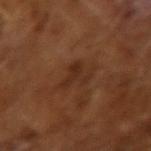This lesion was catalogued during total-body skin photography and was not selected for biopsy. A 15 mm close-up tile from a total-body photography series done for melanoma screening. Measured at roughly 3 mm in maximum diameter. A male patient, in their mid- to late 60s. The lesion-visualizer software estimated a mean CIELAB color near L≈28 a*≈20 b*≈28, roughly 6 lightness units darker than nearby skin, and a lesion-to-skin contrast of about 6 (normalized; higher = more distinct). And it measured peripheral color asymmetry of about 0.5.